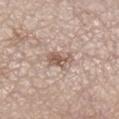<lesion>
<biopsy_status>not biopsied; imaged during a skin examination</biopsy_status>
<automated_metrics>
  <nevus_likeness_0_100>5</nevus_likeness_0_100>
  <lesion_detection_confidence_0_100>100</lesion_detection_confidence_0_100>
</automated_metrics>
<lighting>white-light</lighting>
<site>right lower leg</site>
<patient>
  <sex>female</sex>
  <age_approx>65</age_approx>
</patient>
<image>
  <source>total-body photography crop</source>
  <field_of_view_mm>15</field_of_view_mm>
</image>
<lesion_size>
  <long_diameter_mm_approx>3.0</long_diameter_mm_approx>
</lesion_size>
</lesion>